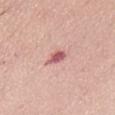No biopsy was performed on this lesion — it was imaged during a full skin examination and was not determined to be concerning. A close-up tile cropped from a whole-body skin photograph, about 15 mm across. A female patient, aged 63 to 67. The tile uses white-light illumination. Approximately 2.5 mm at its widest. On the abdomen.